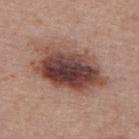Q: Was this lesion biopsied?
A: no biopsy performed (imaged during a skin exam)
Q: What kind of image is this?
A: ~15 mm tile from a whole-body skin photo
Q: How was the tile lit?
A: white-light
Q: Where on the body is the lesion?
A: the upper back
Q: How large is the lesion?
A: about 8.5 mm
Q: Who is the patient?
A: female, aged approximately 50